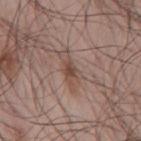<record>
<biopsy_status>not biopsied; imaged during a skin examination</biopsy_status>
<lesion_size>
  <long_diameter_mm_approx>5.0</long_diameter_mm_approx>
</lesion_size>
<automated_metrics>
  <area_mm2_approx>7.0</area_mm2_approx>
  <eccentricity>0.9</eccentricity>
  <shape_asymmetry>0.3</shape_asymmetry>
  <cielab_L>49</cielab_L>
  <cielab_a>17</cielab_a>
  <cielab_b>24</cielab_b>
  <vs_skin_darker_L>8.0</vs_skin_darker_L>
  <vs_skin_contrast_norm>6.0</vs_skin_contrast_norm>
  <nevus_likeness_0_100>0</nevus_likeness_0_100>
  <lesion_detection_confidence_0_100>95</lesion_detection_confidence_0_100>
</automated_metrics>
<patient>
  <sex>male</sex>
  <age_approx>45</age_approx>
</patient>
<image>
  <source>total-body photography crop</source>
  <field_of_view_mm>15</field_of_view_mm>
</image>
<site>back</site>
</record>